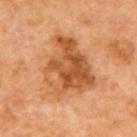follow-up: imaged on a skin check; not biopsied
tile lighting: cross-polarized illumination
acquisition: ~15 mm crop, total-body skin-cancer survey
patient: male, aged around 45
location: the chest
lesion diameter: ~7 mm (longest diameter)